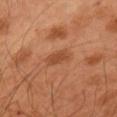• follow-up · catalogued during a skin exam; not biopsied
• image source · ~15 mm crop, total-body skin-cancer survey
• size · ~3 mm (longest diameter)
• illumination · cross-polarized
• body site · the left arm
• subject · male, aged approximately 50